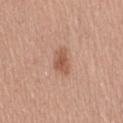Case summary:
* patient — female, about 55 years old
* lesion size — ≈3 mm
* image — ~15 mm tile from a whole-body skin photo
* TBP lesion metrics — a footprint of about 5.5 mm², an eccentricity of roughly 0.75, and two-axis asymmetry of about 0.35; an average lesion color of about L≈55 a*≈23 b*≈30 (CIELAB), roughly 10 lightness units darker than nearby skin, and a lesion-to-skin contrast of about 7 (normalized; higher = more distinct)
* body site — the abdomen
* lighting — white-light illumination